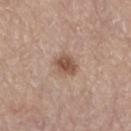follow-up: no biopsy performed (imaged during a skin exam) | lighting: white-light illumination | site: the left lower leg | acquisition: 15 mm crop, total-body photography | patient: female, aged 63 to 67.